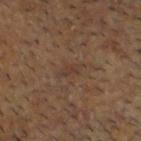{"lesion_size": {"long_diameter_mm_approx": 3.0}, "image": {"source": "total-body photography crop", "field_of_view_mm": 15}, "lighting": "cross-polarized", "site": "head or neck", "patient": {"sex": "male", "age_approx": 60}}